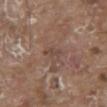Recorded during total-body skin imaging; not selected for excision or biopsy.
From the mid back.
A male patient, roughly 80 years of age.
The tile uses white-light illumination.
This image is a 15 mm lesion crop taken from a total-body photograph.
The recorded lesion diameter is about 2.5 mm.